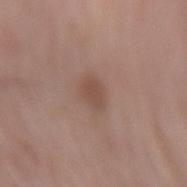{
  "biopsy_status": "not biopsied; imaged during a skin examination",
  "site": "left forearm",
  "image": {
    "source": "total-body photography crop",
    "field_of_view_mm": 15
  },
  "lighting": "white-light",
  "patient": {
    "sex": "male",
    "age_approx": 60
  },
  "lesion_size": {
    "long_diameter_mm_approx": 3.0
  }
}